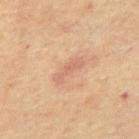Q: Is there a histopathology result?
A: catalogued during a skin exam; not biopsied
Q: How large is the lesion?
A: about 4 mm
Q: How was this image acquired?
A: 15 mm crop, total-body photography
Q: Patient demographics?
A: male, aged 63–67
Q: What lighting was used for the tile?
A: cross-polarized
Q: Where on the body is the lesion?
A: the abdomen
Q: What did automated image analysis measure?
A: an area of roughly 4.5 mm², a shape eccentricity near 0.95, and a symmetry-axis asymmetry near 0.25; a mean CIELAB color near L≈57 a*≈21 b*≈29 and a normalized lesion–skin contrast near 5; border irregularity of about 3.5 on a 0–10 scale and radial color variation of about 0; an automated nevus-likeness rating near 0 out of 100 and lesion-presence confidence of about 100/100